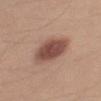No biopsy was performed on this lesion — it was imaged during a full skin examination and was not determined to be concerning. The subject is a male about 20 years old. Automated image analysis of the tile measured a lesion area of about 15 mm². It also reported a lesion–skin lightness drop of about 14 and a normalized border contrast of about 10. And it measured a border-irregularity rating of about 2/10, internal color variation of about 4 on a 0–10 scale, and radial color variation of about 1. And it measured a nevus-likeness score of about 95/100 and a detector confidence of about 100 out of 100 that the crop contains a lesion. The recorded lesion diameter is about 6 mm. A roughly 15 mm field-of-view crop from a total-body skin photograph. Located on the left upper arm. This is a white-light tile.